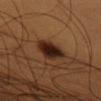biopsy status: catalogued during a skin exam; not biopsied | image: 15 mm crop, total-body photography | subject: male, aged around 60 | site: the front of the torso.